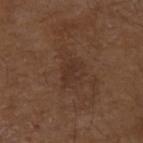Captured during whole-body skin photography for melanoma surveillance; the lesion was not biopsied.
A male subject, aged around 75.
A lesion tile, about 15 mm wide, cut from a 3D total-body photograph.
From the right upper arm.